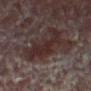Clinical impression:
No biopsy was performed on this lesion — it was imaged during a full skin examination and was not determined to be concerning.
Background:
A male patient aged around 55. Cropped from a whole-body photographic skin survey; the tile spans about 15 mm. The lesion is located on the left thigh. The tile uses cross-polarized illumination. About 6.5 mm across. Automated image analysis of the tile measured a mean CIELAB color near L≈23 a*≈14 b*≈15 and a lesion–skin lightness drop of about 6. The analysis additionally found a border-irregularity index near 6.5/10 and a peripheral color-asymmetry measure near 1. The analysis additionally found a nevus-likeness score of about 5/100 and a detector confidence of about 75 out of 100 that the crop contains a lesion.